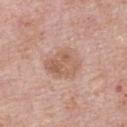Notes:
- biopsy status · catalogued during a skin exam; not biopsied
- site · the right upper arm
- subject · male, aged 73–77
- diameter · ≈4.5 mm
- acquisition · ~15 mm tile from a whole-body skin photo